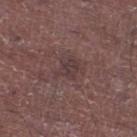Assessment:
Imaged during a routine full-body skin examination; the lesion was not biopsied and no histopathology is available.
Clinical summary:
Automated tile analysis of the lesion measured a footprint of about 7 mm², an outline eccentricity of about 0.6 (0 = round, 1 = elongated), and a shape-asymmetry score of about 0.4 (0 = symmetric). The analysis additionally found an average lesion color of about L≈37 a*≈17 b*≈16 (CIELAB), a lesion–skin lightness drop of about 6, and a lesion-to-skin contrast of about 6 (normalized; higher = more distinct). The software also gave a border-irregularity index near 4.5/10, a color-variation rating of about 2.5/10, and peripheral color asymmetry of about 1. This is a white-light tile. The recorded lesion diameter is about 3.5 mm. From the right lower leg. A male patient, about 65 years old. This image is a 15 mm lesion crop taken from a total-body photograph.